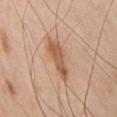Part of a total-body skin-imaging series; this lesion was reviewed on a skin check and was not flagged for biopsy.
From the chest.
A 15 mm crop from a total-body photograph taken for skin-cancer surveillance.
A male subject, in their 60s.
This is a white-light tile.
About 5.5 mm across.
Automated image analysis of the tile measured an area of roughly 9 mm², a shape eccentricity near 0.95, and a shape-asymmetry score of about 0.3 (0 = symmetric). The software also gave a mean CIELAB color near L≈58 a*≈21 b*≈33 and a lesion-to-skin contrast of about 7.5 (normalized; higher = more distinct). The software also gave a within-lesion color-variation index near 3/10. It also reported an automated nevus-likeness rating near 35 out of 100 and a detector confidence of about 100 out of 100 that the crop contains a lesion.